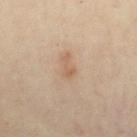Assessment: Captured during whole-body skin photography for melanoma surveillance; the lesion was not biopsied. Background: Automated tile analysis of the lesion measured a lesion area of about 3 mm², an eccentricity of roughly 0.85, and a shape-asymmetry score of about 0.55 (0 = symmetric). The patient is a female in their 30s. Located on the chest. Measured at roughly 2.5 mm in maximum diameter. Imaged with cross-polarized lighting. A region of skin cropped from a whole-body photographic capture, roughly 15 mm wide.